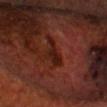Part of a total-body skin-imaging series; this lesion was reviewed on a skin check and was not flagged for biopsy.
The subject is a male aged 68–72.
Captured under cross-polarized illumination.
The lesion-visualizer software estimated about 7 CIELAB-L* units darker than the surrounding skin and a normalized border contrast of about 7.5. And it measured internal color variation of about 2.5 on a 0–10 scale and a peripheral color-asymmetry measure near 1. And it measured a nevus-likeness score of about 0/100 and lesion-presence confidence of about 60/100.
Cropped from a whole-body photographic skin survey; the tile spans about 15 mm.
Approximately 4 mm at its widest.
Located on the head or neck.